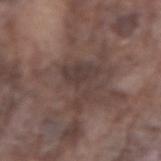Imaged during a routine full-body skin examination; the lesion was not biopsied and no histopathology is available. From the arm. A male patient roughly 75 years of age. The lesion's longest dimension is about 5 mm. Automated tile analysis of the lesion measured an area of roughly 10 mm² and a symmetry-axis asymmetry near 0.6. The analysis additionally found internal color variation of about 2.5 on a 0–10 scale and peripheral color asymmetry of about 1. Captured under white-light illumination. A region of skin cropped from a whole-body photographic capture, roughly 15 mm wide.